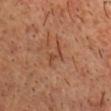Assessment: The lesion was tiled from a total-body skin photograph and was not biopsied. Acquisition and patient details: Imaged with cross-polarized lighting. Automated tile analysis of the lesion measured a classifier nevus-likeness of about 0/100 and lesion-presence confidence of about 100/100. A male patient, aged 53 to 57. Approximately 2.5 mm at its widest. From the chest. Cropped from a total-body skin-imaging series; the visible field is about 15 mm.